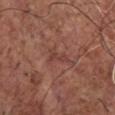Impression:
Recorded during total-body skin imaging; not selected for excision or biopsy.
Background:
The lesion is located on the chest. About 2.5 mm across. The patient is a male roughly 80 years of age. A region of skin cropped from a whole-body photographic capture, roughly 15 mm wide. Automated image analysis of the tile measured a mean CIELAB color near L≈41 a*≈24 b*≈25. The software also gave peripheral color asymmetry of about 0.